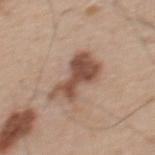Part of a total-body skin-imaging series; this lesion was reviewed on a skin check and was not flagged for biopsy. This image is a 15 mm lesion crop taken from a total-body photograph. The lesion's longest dimension is about 6 mm. The lesion is located on the back. This is a white-light tile. A male subject, aged approximately 65.